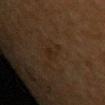Findings:
– workup: total-body-photography surveillance lesion; no biopsy
– patient: female, aged 38 to 42
– diameter: about 3.5 mm
– TBP lesion metrics: a lesion area of about 3 mm², a shape eccentricity near 0.9, and a shape-asymmetry score of about 0.4 (0 = symmetric); a mean CIELAB color near L≈19 a*≈12 b*≈21, a lesion–skin lightness drop of about 4, and a lesion-to-skin contrast of about 5.5 (normalized; higher = more distinct); border irregularity of about 5 on a 0–10 scale, a color-variation rating of about 1/10, and radial color variation of about 0.5; an automated nevus-likeness rating near 0 out of 100
– body site: the chest
– image: ~15 mm tile from a whole-body skin photo
– lighting: cross-polarized